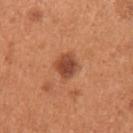This lesion was catalogued during total-body skin photography and was not selected for biopsy. The lesion is located on the left upper arm. Cropped from a whole-body photographic skin survey; the tile spans about 15 mm. A female subject approximately 30 years of age.